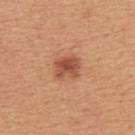The lesion was photographed on a routine skin check and not biopsied; there is no pathology result. The subject is a male roughly 55 years of age. The tile uses white-light illumination. The lesion-visualizer software estimated a border-irregularity rating of about 1.5/10 and a within-lesion color-variation index near 4/10. The analysis additionally found an automated nevus-likeness rating near 85 out of 100 and a lesion-detection confidence of about 100/100. Measured at roughly 3.5 mm in maximum diameter. A 15 mm close-up extracted from a 3D total-body photography capture. From the back.